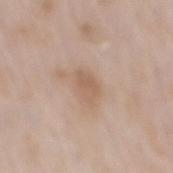{
  "automated_metrics": {
    "eccentricity": 0.75,
    "shape_asymmetry": 0.2,
    "vs_skin_darker_L": 8.0,
    "vs_skin_contrast_norm": 5.5,
    "nevus_likeness_0_100": 5,
    "lesion_detection_confidence_0_100": 100
  },
  "patient": {
    "sex": "male",
    "age_approx": 65
  },
  "site": "mid back",
  "image": {
    "source": "total-body photography crop",
    "field_of_view_mm": 15
  }
}